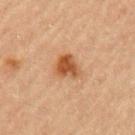The lesion is on the left upper arm.
This image is a 15 mm lesion crop taken from a total-body photograph.
Measured at roughly 3 mm in maximum diameter.
Automated image analysis of the tile measured internal color variation of about 3 on a 0–10 scale and a peripheral color-asymmetry measure near 1.
This is a cross-polarized tile.
The patient is a female in their mid- to late 60s.